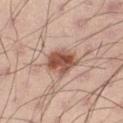automated_metrics:
  lesion_detection_confidence_0_100: 100
site: right thigh
patient:
  sex: male
  age_approx: 45
lesion_size:
  long_diameter_mm_approx: 4.0
lighting: white-light
image:
  source: total-body photography crop
  field_of_view_mm: 15